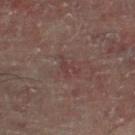Assessment: Recorded during total-body skin imaging; not selected for excision or biopsy. Context: Cropped from a total-body skin-imaging series; the visible field is about 15 mm. Approximately 3 mm at its widest. A male patient, aged around 60. The lesion is on the right lower leg. Imaged with cross-polarized lighting. An algorithmic analysis of the crop reported a lesion area of about 3 mm² and two-axis asymmetry of about 0.7. And it measured a mean CIELAB color near L≈33 a*≈18 b*≈16, about 5 CIELAB-L* units darker than the surrounding skin, and a normalized lesion–skin contrast near 4.5. The software also gave a border-irregularity index near 8.5/10, a color-variation rating of about 0/10, and a peripheral color-asymmetry measure near 0.